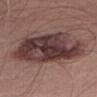Captured during whole-body skin photography for melanoma surveillance; the lesion was not biopsied. A 15 mm close-up extracted from a 3D total-body photography capture. A male subject aged 58 to 62. On the right thigh. Imaged with white-light lighting.